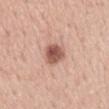follow-up — catalogued during a skin exam; not biopsied
patient — male, aged around 55
anatomic site — the mid back
image source — ~15 mm tile from a whole-body skin photo
lighting — white-light